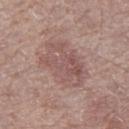The lesion was photographed on a routine skin check and not biopsied; there is no pathology result. The lesion is on the leg. A male subject, approximately 70 years of age. This image is a 15 mm lesion crop taken from a total-body photograph. The lesion-visualizer software estimated a footprint of about 21 mm², an outline eccentricity of about 0.7 (0 = round, 1 = elongated), and two-axis asymmetry of about 0.2. The analysis additionally found a lesion color around L≈53 a*≈19 b*≈21 in CIELAB, a lesion–skin lightness drop of about 8, and a lesion-to-skin contrast of about 5.5 (normalized; higher = more distinct). It also reported a border-irregularity rating of about 2.5/10, internal color variation of about 4.5 on a 0–10 scale, and a peripheral color-asymmetry measure near 1.5.